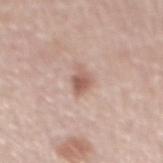biopsy status=imaged on a skin check; not biopsied | subject=male, aged approximately 60 | imaging modality=~15 mm tile from a whole-body skin photo | body site=the back | lighting=white-light | lesion size=≈3 mm | automated metrics=a lesion–skin lightness drop of about 11 and a normalized lesion–skin contrast near 7.5; a border-irregularity index near 3.5/10 and internal color variation of about 3.5 on a 0–10 scale; an automated nevus-likeness rating near 60 out of 100 and a detector confidence of about 100 out of 100 that the crop contains a lesion.